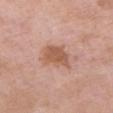This lesion was catalogued during total-body skin photography and was not selected for biopsy.
The lesion-visualizer software estimated a mean CIELAB color near L≈57 a*≈22 b*≈31 and roughly 10 lightness units darker than nearby skin. The analysis additionally found a peripheral color-asymmetry measure near 0.5. The analysis additionally found a classifier nevus-likeness of about 35/100 and lesion-presence confidence of about 100/100.
The lesion is located on the chest.
Imaged with white-light lighting.
Approximately 4 mm at its widest.
A 15 mm close-up tile from a total-body photography series done for melanoma screening.
The patient is a female approximately 70 years of age.